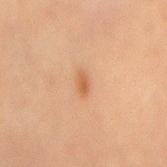{
  "biopsy_status": "not biopsied; imaged during a skin examination",
  "patient": {
    "sex": "male",
    "age_approx": 70
  },
  "image": {
    "source": "total-body photography crop",
    "field_of_view_mm": 15
  },
  "site": "abdomen"
}